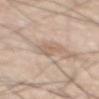Clinical summary: The lesion is on the mid back. A 15 mm crop from a total-body photograph taken for skin-cancer surveillance. The subject is a male roughly 65 years of age. The tile uses white-light illumination. Approximately 3 mm at its widest.